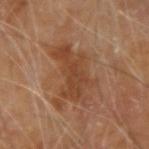Captured during whole-body skin photography for melanoma surveillance; the lesion was not biopsied.
Measured at roughly 7 mm in maximum diameter.
The lesion is on the right forearm.
Imaged with cross-polarized lighting.
This image is a 15 mm lesion crop taken from a total-body photograph.
A male subject roughly 70 years of age.